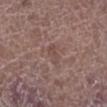Assessment:
The lesion was photographed on a routine skin check and not biopsied; there is no pathology result.
Context:
A male patient, in their 70s. The lesion's longest dimension is about 2.5 mm. The lesion-visualizer software estimated a border-irregularity rating of about 5/10, internal color variation of about 0 on a 0–10 scale, and a peripheral color-asymmetry measure near 0. The analysis additionally found an automated nevus-likeness rating near 0 out of 100 and a lesion-detection confidence of about 95/100. A 15 mm close-up extracted from a 3D total-body photography capture. Imaged with white-light lighting. From the left lower leg.